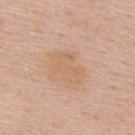This lesion was catalogued during total-body skin photography and was not selected for biopsy. Longest diameter approximately 4.5 mm. Automated image analysis of the tile measured a lesion area of about 10 mm², an outline eccentricity of about 0.8 (0 = round, 1 = elongated), and two-axis asymmetry of about 0.3. And it measured a classifier nevus-likeness of about 0/100. Cropped from a total-body skin-imaging series; the visible field is about 15 mm. The lesion is located on the upper back. The patient is a male approximately 55 years of age. Captured under white-light illumination.